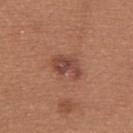Q: Was a biopsy performed?
A: total-body-photography surveillance lesion; no biopsy
Q: What are the patient's age and sex?
A: female, approximately 25 years of age
Q: How was this image acquired?
A: ~15 mm tile from a whole-body skin photo
Q: Where on the body is the lesion?
A: the upper back
Q: How large is the lesion?
A: about 3.5 mm